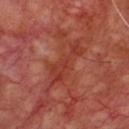On the chest. This is a cross-polarized tile. A close-up tile cropped from a whole-body skin photograph, about 15 mm across. Measured at roughly 6 mm in maximum diameter. A male subject aged approximately 70.